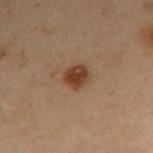* follow-up — imaged on a skin check; not biopsied
* anatomic site — the right upper arm
* subject — male, aged approximately 55
* lesion diameter — ~2.5 mm (longest diameter)
* image — ~15 mm tile from a whole-body skin photo
* automated lesion analysis — a lesion color around L≈32 a*≈17 b*≈27 in CIELAB and a lesion–skin lightness drop of about 10